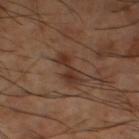{"biopsy_status": "not biopsied; imaged during a skin examination", "image": {"source": "total-body photography crop", "field_of_view_mm": 15}, "patient": {"sex": "male", "age_approx": 65}, "site": "leg", "lesion_size": {"long_diameter_mm_approx": 4.0}, "lighting": "cross-polarized"}